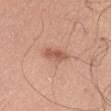| key | value |
|---|---|
| notes | no biopsy performed (imaged during a skin exam) |
| image-analysis metrics | a lesion–skin lightness drop of about 11 and a normalized border contrast of about 7 |
| size | ~3.5 mm (longest diameter) |
| image | total-body-photography crop, ~15 mm field of view |
| subject | male, roughly 40 years of age |
| location | the left upper arm |
| tile lighting | white-light |